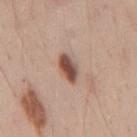- workup: total-body-photography surveillance lesion; no biopsy
- image: ~15 mm crop, total-body skin-cancer survey
- site: the mid back
- patient: male, in their 40s
- tile lighting: white-light